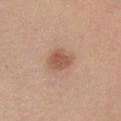{"biopsy_status": "not biopsied; imaged during a skin examination"}